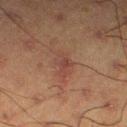  biopsy_status: not biopsied; imaged during a skin examination
  lighting: cross-polarized
  patient:
    sex: male
    age_approx: 60
  lesion_size:
    long_diameter_mm_approx: 3.5
  image:
    source: total-body photography crop
    field_of_view_mm: 15
  site: left thigh
  automated_metrics:
    area_mm2_approx: 5.0
    eccentricity: 0.85
    shape_asymmetry: 0.35
    vs_skin_darker_L: 5.0
    vs_skin_contrast_norm: 5.5
    border_irregularity_0_10: 4.0
    color_variation_0_10: 2.5
    peripheral_color_asymmetry: 0.5